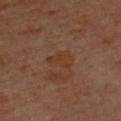  biopsy_status: not biopsied; imaged during a skin examination